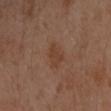{"biopsy_status": "not biopsied; imaged during a skin examination", "patient": {"sex": "female", "age_approx": 40}, "site": "left forearm", "lighting": "cross-polarized", "image": {"source": "total-body photography crop", "field_of_view_mm": 15}, "lesion_size": {"long_diameter_mm_approx": 3.5}}